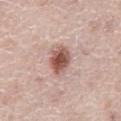– biopsy status · catalogued during a skin exam; not biopsied
– image-analysis metrics · a lesion area of about 7.5 mm², an outline eccentricity of about 0.7 (0 = round, 1 = elongated), and a shape-asymmetry score of about 0.2 (0 = symmetric); a lesion color around L≈54 a*≈21 b*≈26 in CIELAB, roughly 16 lightness units darker than nearby skin, and a normalized lesion–skin contrast near 10.5; internal color variation of about 4.5 on a 0–10 scale and peripheral color asymmetry of about 1; a nevus-likeness score of about 95/100 and a lesion-detection confidence of about 100/100
– lesion size · about 3.5 mm
– subject · male, aged 73 to 77
– tile lighting · white-light
– location · the abdomen
– image · total-body-photography crop, ~15 mm field of view